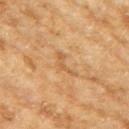| feature | finding |
|---|---|
| biopsy status | total-body-photography surveillance lesion; no biopsy |
| imaging modality | ~15 mm tile from a whole-body skin photo |
| anatomic site | the right upper arm |
| subject | female, about 60 years old |